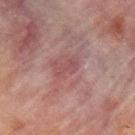lesion size = ≈3 mm
acquisition = 15 mm crop, total-body photography
tile lighting = cross-polarized
location = the upper back
automated lesion analysis = a classifier nevus-likeness of about 0/100 and a detector confidence of about 100 out of 100 that the crop contains a lesion
subject = male, in their 70s